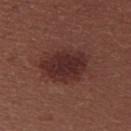Clinical impression:
Recorded during total-body skin imaging; not selected for excision or biopsy.
Image and clinical context:
A female subject aged 23–27. This image is a 15 mm lesion crop taken from a total-body photograph. The lesion is on the back.